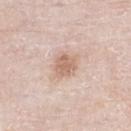Part of a total-body skin-imaging series; this lesion was reviewed on a skin check and was not flagged for biopsy.
A region of skin cropped from a whole-body photographic capture, roughly 15 mm wide.
About 3 mm across.
The subject is a male aged approximately 80.
The lesion-visualizer software estimated a lesion color around L≈65 a*≈18 b*≈28 in CIELAB, a lesion–skin lightness drop of about 11, and a lesion-to-skin contrast of about 7 (normalized; higher = more distinct). And it measured a nevus-likeness score of about 15/100 and a detector confidence of about 100 out of 100 that the crop contains a lesion.
The lesion is located on the left lower leg.
This is a white-light tile.